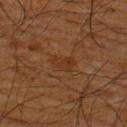<case>
<biopsy_status>not biopsied; imaged during a skin examination</biopsy_status>
<lighting>cross-polarized</lighting>
<automated_metrics>
  <area_mm2_approx>4.5</area_mm2_approx>
  <shape_asymmetry>0.3</shape_asymmetry>
  <border_irregularity_0_10>3.0</border_irregularity_0_10>
  <color_variation_0_10>2.0</color_variation_0_10>
  <peripheral_color_asymmetry>0.5</peripheral_color_asymmetry>
  <nevus_likeness_0_100>0</nevus_likeness_0_100>
  <lesion_detection_confidence_0_100>100</lesion_detection_confidence_0_100>
</automated_metrics>
<lesion_size>
  <long_diameter_mm_approx>3.0</long_diameter_mm_approx>
</lesion_size>
<site>upper back</site>
<image>
  <source>total-body photography crop</source>
  <field_of_view_mm>15</field_of_view_mm>
</image>
<patient>
  <sex>male</sex>
  <age_approx>65</age_approx>
</patient>
</case>